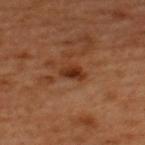| feature | finding |
|---|---|
| follow-up | imaged on a skin check; not biopsied |
| automated metrics | a footprint of about 3.5 mm², a shape eccentricity near 0.85, and a symmetry-axis asymmetry near 0.25; border irregularity of about 2 on a 0–10 scale, internal color variation of about 2.5 on a 0–10 scale, and peripheral color asymmetry of about 1; a detector confidence of about 100 out of 100 that the crop contains a lesion |
| site | the upper back |
| patient | male, aged around 50 |
| image source | 15 mm crop, total-body photography |
| size | ~3 mm (longest diameter) |
| lighting | cross-polarized |